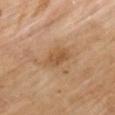Recorded during total-body skin imaging; not selected for excision or biopsy. Imaged with cross-polarized lighting. The lesion-visualizer software estimated an automated nevus-likeness rating near 0 out of 100 and a lesion-detection confidence of about 100/100. The lesion is located on the left upper arm. A region of skin cropped from a whole-body photographic capture, roughly 15 mm wide. A male patient, about 70 years old.